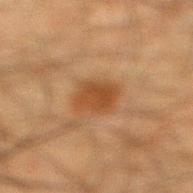notes = imaged on a skin check; not biopsied | acquisition = ~15 mm tile from a whole-body skin photo | lesion diameter = ≈3 mm | body site = the right lower leg | patient = male, about 35 years old | illumination = cross-polarized illumination.